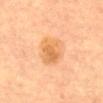workup: no biopsy performed (imaged during a skin exam) | illumination: cross-polarized illumination | image: 15 mm crop, total-body photography | size: ≈4.5 mm | automated lesion analysis: a footprint of about 12 mm² and an outline eccentricity of about 0.7 (0 = round, 1 = elongated); a classifier nevus-likeness of about 25/100 | patient: female, aged 58–62 | body site: the abdomen.